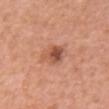{"biopsy_status": "not biopsied; imaged during a skin examination", "automated_metrics": {"area_mm2_approx": 5.5, "eccentricity": 0.65, "shape_asymmetry": 0.25, "border_irregularity_0_10": 3.0, "color_variation_0_10": 4.0, "peripheral_color_asymmetry": 1.0, "lesion_detection_confidence_0_100": 100}, "site": "chest", "image": {"source": "total-body photography crop", "field_of_view_mm": 15}, "patient": {"sex": "female", "age_approx": 40}, "lesion_size": {"long_diameter_mm_approx": 3.0}}